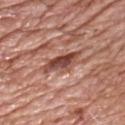Background:
Cropped from a total-body skin-imaging series; the visible field is about 15 mm. The lesion is on the chest. The subject is a male aged 58 to 62. This is a white-light tile.